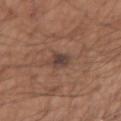Impression: The lesion was photographed on a routine skin check and not biopsied; there is no pathology result. Clinical summary: This image is a 15 mm lesion crop taken from a total-body photograph. The lesion is on the right upper arm. Captured under white-light illumination. The subject is a male in their mid-50s.